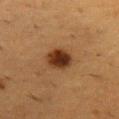follow-up: imaged on a skin check; not biopsied
illumination: cross-polarized
imaging modality: 15 mm crop, total-body photography
patient: female, aged around 55
location: the chest
TBP lesion metrics: an area of roughly 7.5 mm² and a shape eccentricity near 0.45; an average lesion color of about L≈28 a*≈18 b*≈28 (CIELAB) and about 13 CIELAB-L* units darker than the surrounding skin